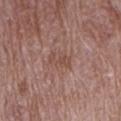biopsy_status: not biopsied; imaged during a skin examination
image:
  source: total-body photography crop
  field_of_view_mm: 15
site: arm
lesion_size:
  long_diameter_mm_approx: 3.0
patient:
  sex: female
  age_approx: 70